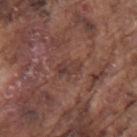Assessment: Part of a total-body skin-imaging series; this lesion was reviewed on a skin check and was not flagged for biopsy. Image and clinical context: A roughly 15 mm field-of-view crop from a total-body skin photograph. A male patient in their mid- to late 70s. The lesion-visualizer software estimated an average lesion color of about L≈38 a*≈19 b*≈22 (CIELAB), roughly 6 lightness units darker than nearby skin, and a normalized border contrast of about 5.5. And it measured a nevus-likeness score of about 0/100. The lesion is located on the left upper arm. Measured at roughly 3 mm in maximum diameter. Imaged with white-light lighting.